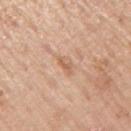  patient:
    sex: male
    age_approx: 75
  image:
    source: total-body photography crop
    field_of_view_mm: 15
  site: arm